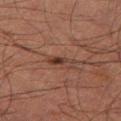The lesion was tiled from a total-body skin photograph and was not biopsied.
The lesion is on the left thigh.
Imaged with cross-polarized lighting.
The recorded lesion diameter is about 3 mm.
A male subject, approximately 50 years of age.
Automated tile analysis of the lesion measured a footprint of about 3 mm² and a symmetry-axis asymmetry near 0.35. The software also gave a lesion color around L≈28 a*≈16 b*≈21 in CIELAB, roughly 8 lightness units darker than nearby skin, and a normalized lesion–skin contrast near 8. The analysis additionally found a nevus-likeness score of about 75/100 and a lesion-detection confidence of about 100/100.
A 15 mm close-up extracted from a 3D total-body photography capture.